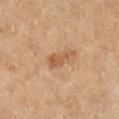Clinical impression: Part of a total-body skin-imaging series; this lesion was reviewed on a skin check and was not flagged for biopsy. Image and clinical context: The tile uses cross-polarized illumination. The lesion's longest dimension is about 4 mm. An algorithmic analysis of the crop reported a border-irregularity rating of about 5/10, internal color variation of about 2.5 on a 0–10 scale, and peripheral color asymmetry of about 0.5. The lesion is located on the right lower leg. A 15 mm crop from a total-body photograph taken for skin-cancer surveillance. A female subject, in their 60s.